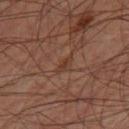Clinical impression: This lesion was catalogued during total-body skin photography and was not selected for biopsy. Image and clinical context: A close-up tile cropped from a whole-body skin photograph, about 15 mm across. Automated image analysis of the tile measured a lesion color around L≈32 a*≈18 b*≈25 in CIELAB, about 6 CIELAB-L* units darker than the surrounding skin, and a normalized border contrast of about 6. On the left thigh. This is a cross-polarized tile. A male patient in their 60s.